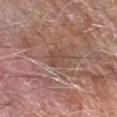The lesion was tiled from a total-body skin photograph and was not biopsied.
From the right forearm.
This image is a 15 mm lesion crop taken from a total-body photograph.
The total-body-photography lesion software estimated an area of roughly 5.5 mm², a shape eccentricity near 0.6, and a shape-asymmetry score of about 0.3 (0 = symmetric). The software also gave a mean CIELAB color near L≈48 a*≈19 b*≈26 and a normalized border contrast of about 5. And it measured a border-irregularity rating of about 3/10 and a color-variation rating of about 2.5/10. And it measured a detector confidence of about 75 out of 100 that the crop contains a lesion.
The patient is a male in their mid-70s.
The recorded lesion diameter is about 3 mm.
The tile uses white-light illumination.